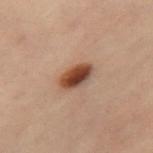Notes:
• workup: total-body-photography surveillance lesion; no biopsy
• lesion diameter: ≈4 mm
• image: 15 mm crop, total-body photography
• site: the left leg
• subject: female, roughly 60 years of age